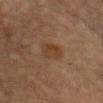The lesion was photographed on a routine skin check and not biopsied; there is no pathology result.